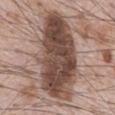Assessment:
Captured during whole-body skin photography for melanoma surveillance; the lesion was not biopsied.
Context:
A close-up tile cropped from a whole-body skin photograph, about 15 mm across. The tile uses white-light illumination. The lesion is on the abdomen. A male subject, about 70 years old. Automated image analysis of the tile measured an automated nevus-likeness rating near 35 out of 100 and lesion-presence confidence of about 100/100.